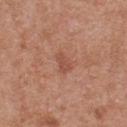Image and clinical context: The lesion is located on the upper back. This is a white-light tile. The total-body-photography lesion software estimated an outline eccentricity of about 0.7 (0 = round, 1 = elongated) and a shape-asymmetry score of about 0.3 (0 = symmetric). The software also gave a mean CIELAB color near L≈51 a*≈25 b*≈30, a lesion–skin lightness drop of about 7, and a normalized border contrast of about 5.5. The analysis additionally found a lesion-detection confidence of about 100/100. A male patient, in their 30s. A 15 mm close-up extracted from a 3D total-body photography capture. The lesion's longest dimension is about 2.5 mm.